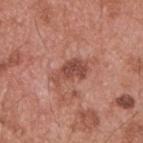<case>
  <biopsy_status>not biopsied; imaged during a skin examination</biopsy_status>
  <patient>
    <sex>male</sex>
    <age_approx>55</age_approx>
  </patient>
  <image>
    <source>total-body photography crop</source>
    <field_of_view_mm>15</field_of_view_mm>
  </image>
  <lesion_size>
    <long_diameter_mm_approx>4.0</long_diameter_mm_approx>
  </lesion_size>
  <lighting>white-light</lighting>
  <site>upper back</site>
</case>